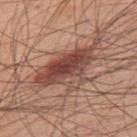notes — total-body-photography surveillance lesion; no biopsy
diameter — ~8.5 mm (longest diameter)
image source — 15 mm crop, total-body photography
site — the upper back
illumination — white-light
subject — male, aged 58 to 62